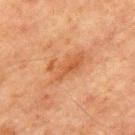Assessment:
The lesion was tiled from a total-body skin photograph and was not biopsied.
Context:
Imaged with cross-polarized lighting. A male patient aged approximately 65. Cropped from a total-body skin-imaging series; the visible field is about 15 mm. The lesion's longest dimension is about 4.5 mm. On the chest. An algorithmic analysis of the crop reported an average lesion color of about L≈45 a*≈22 b*≈34 (CIELAB), about 7 CIELAB-L* units darker than the surrounding skin, and a normalized lesion–skin contrast near 6. And it measured a border-irregularity rating of about 6/10, internal color variation of about 3 on a 0–10 scale, and a peripheral color-asymmetry measure near 1.